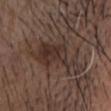The lesion was photographed on a routine skin check and not biopsied; there is no pathology result. The lesion is on the head or neck. The tile uses white-light illumination. A 15 mm close-up tile from a total-body photography series done for melanoma screening. Approximately 7.5 mm at its widest. A male patient, aged around 75.